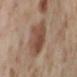subject = female, about 55 years old; image source = ~15 mm tile from a whole-body skin photo; anatomic site = the left lower leg.